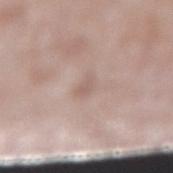| field | value |
|---|---|
| follow-up | total-body-photography surveillance lesion; no biopsy |
| diameter | about 3 mm |
| imaging modality | ~15 mm tile from a whole-body skin photo |
| subject | male, roughly 60 years of age |
| anatomic site | the right lower leg |
| tile lighting | white-light illumination |